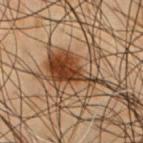workup: total-body-photography surveillance lesion; no biopsy | diameter: ~8 mm (longest diameter) | lighting: cross-polarized | automated lesion analysis: border irregularity of about 9 on a 0–10 scale and a peripheral color-asymmetry measure near 2 | patient: male, in their mid-50s | acquisition: ~15 mm tile from a whole-body skin photo | body site: the chest.